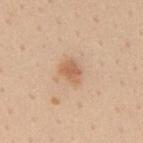{"biopsy_status": "not biopsied; imaged during a skin examination", "automated_metrics": {"area_mm2_approx": 4.0, "eccentricity": 0.65, "shape_asymmetry": 0.3}, "site": "mid back", "patient": {"sex": "female", "age_approx": 45}, "lesion_size": {"long_diameter_mm_approx": 2.5}, "image": {"source": "total-body photography crop", "field_of_view_mm": 15}, "lighting": "white-light"}